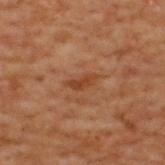Longest diameter approximately 3 mm.
Captured under cross-polarized illumination.
Cropped from a whole-body photographic skin survey; the tile spans about 15 mm.
Located on the upper back.
The subject is a male about 70 years old.